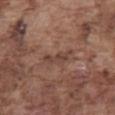follow-up: no biopsy performed (imaged during a skin exam); diameter: ~4 mm (longest diameter); body site: the abdomen; illumination: white-light; image: 15 mm crop, total-body photography; patient: male, aged 73–77.